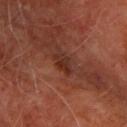Part of a total-body skin-imaging series; this lesion was reviewed on a skin check and was not flagged for biopsy. From the upper back. A 15 mm crop from a total-body photograph taken for skin-cancer surveillance. Measured at roughly 3 mm in maximum diameter. The tile uses cross-polarized illumination. A male subject, aged approximately 65.